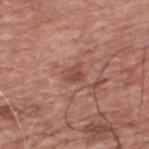This lesion was catalogued during total-body skin photography and was not selected for biopsy.
The tile uses white-light illumination.
A lesion tile, about 15 mm wide, cut from a 3D total-body photograph.
Approximately 2.5 mm at its widest.
From the upper back.
The patient is a male aged 58–62.